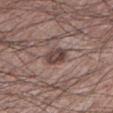A close-up tile cropped from a whole-body skin photograph, about 15 mm across.
This is a white-light tile.
Measured at roughly 3 mm in maximum diameter.
From the left lower leg.
The subject is a male roughly 60 years of age.